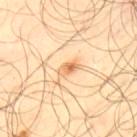  patient:
    sex: male
    age_approx: 65
  lesion_size:
    long_diameter_mm_approx: 2.5
  lighting: cross-polarized
  site: upper back
  automated_metrics:
    area_mm2_approx: 3.0
    eccentricity: 0.85
    shape_asymmetry: 0.3
    cielab_L: 67
    cielab_a: 21
    cielab_b: 41
    vs_skin_contrast_norm: 7.5
    border_irregularity_0_10: 2.5
    color_variation_0_10: 6.5
    peripheral_color_asymmetry: 3.0
  image:
    source: total-body photography crop
    field_of_view_mm: 15